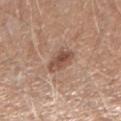Case summary:
• notes — catalogued during a skin exam; not biopsied
• subject — female, about 60 years old
• illumination — white-light illumination
• diameter — about 3 mm
• body site — the right forearm
• imaging modality — total-body-photography crop, ~15 mm field of view